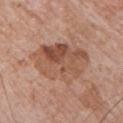{"biopsy_status": "not biopsied; imaged during a skin examination", "patient": {"sex": "male", "age_approx": 70}, "site": "chest", "lighting": "white-light", "lesion_size": {"long_diameter_mm_approx": 7.5}, "image": {"source": "total-body photography crop", "field_of_view_mm": 15}}